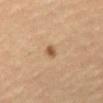{"biopsy_status": "not biopsied; imaged during a skin examination", "lesion_size": {"long_diameter_mm_approx": 2.0}, "site": "abdomen", "patient": {"sex": "female", "age_approx": 65}, "automated_metrics": {"area_mm2_approx": 2.0, "eccentricity": 0.8, "vs_skin_contrast_norm": 8.5, "nevus_likeness_0_100": 95, "lesion_detection_confidence_0_100": 100}, "image": {"source": "total-body photography crop", "field_of_view_mm": 15}}